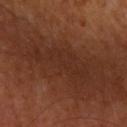Captured during whole-body skin photography for melanoma surveillance; the lesion was not biopsied.
The lesion is on the left upper arm.
Automated tile analysis of the lesion measured a border-irregularity rating of about 7/10 and peripheral color asymmetry of about 0.5.
The patient is a male aged 58–62.
This image is a 15 mm lesion crop taken from a total-body photograph.
This is a cross-polarized tile.
About 7 mm across.